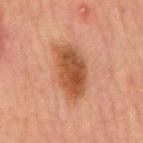Impression: This lesion was catalogued during total-body skin photography and was not selected for biopsy. Background: Imaged with cross-polarized lighting. A male patient in their mid- to late 60s. From the front of the torso. A region of skin cropped from a whole-body photographic capture, roughly 15 mm wide. The lesion-visualizer software estimated a footprint of about 16 mm² and two-axis asymmetry of about 0.2. The analysis additionally found a nevus-likeness score of about 90/100 and a detector confidence of about 100 out of 100 that the crop contains a lesion.